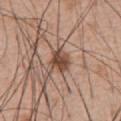Assessment:
No biopsy was performed on this lesion — it was imaged during a full skin examination and was not determined to be concerning.
Clinical summary:
A male patient aged approximately 55. The recorded lesion diameter is about 3 mm. Imaged with white-light lighting. An algorithmic analysis of the crop reported a lesion color around L≈47 a*≈20 b*≈28 in CIELAB, a lesion–skin lightness drop of about 13, and a lesion-to-skin contrast of about 9.5 (normalized; higher = more distinct). The analysis additionally found a classifier nevus-likeness of about 85/100 and a detector confidence of about 100 out of 100 that the crop contains a lesion. A 15 mm close-up tile from a total-body photography series done for melanoma screening. Located on the abdomen.